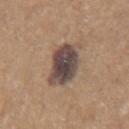Q: Lesion size?
A: ~5 mm (longest diameter)
Q: Lesion location?
A: the left upper arm
Q: Patient demographics?
A: male, aged 63–67
Q: What kind of image is this?
A: ~15 mm crop, total-body skin-cancer survey